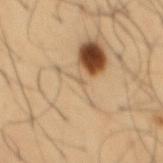Q: Is there a histopathology result?
A: imaged on a skin check; not biopsied
Q: Automated lesion metrics?
A: an outline eccentricity of about 0.7 (0 = round, 1 = elongated) and a symmetry-axis asymmetry near 0.35; border irregularity of about 3 on a 0–10 scale, a color-variation rating of about 0/10, and a peripheral color-asymmetry measure near 0; lesion-presence confidence of about 0/100
Q: Patient demographics?
A: male, in their 40s
Q: What kind of image is this?
A: ~15 mm crop, total-body skin-cancer survey
Q: Lesion size?
A: about 1 mm
Q: Where on the body is the lesion?
A: the mid back